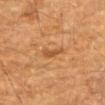Assessment:
Imaged during a routine full-body skin examination; the lesion was not biopsied and no histopathology is available.
Context:
This image is a 15 mm lesion crop taken from a total-body photograph. From the arm. A male subject roughly 85 years of age or older.